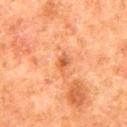workup=total-body-photography surveillance lesion; no biopsy
TBP lesion metrics=a border-irregularity index near 3/10, internal color variation of about 5 on a 0–10 scale, and a peripheral color-asymmetry measure near 1.5; a nevus-likeness score of about 0/100 and a lesion-detection confidence of about 100/100
patient=male, aged around 80
lesion size=about 3 mm
acquisition=total-body-photography crop, ~15 mm field of view
site=the mid back
tile lighting=cross-polarized